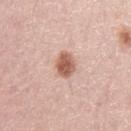Q: Is there a histopathology result?
A: no biopsy performed (imaged during a skin exam)
Q: What is the anatomic site?
A: the left upper arm
Q: Lesion size?
A: ≈3.5 mm
Q: What lighting was used for the tile?
A: white-light
Q: What did automated image analysis measure?
A: an area of roughly 6 mm² and a shape-asymmetry score of about 0.2 (0 = symmetric)
Q: Who is the patient?
A: male, in their mid-40s
Q: What is the imaging modality?
A: total-body-photography crop, ~15 mm field of view